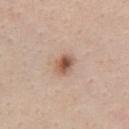Part of a total-body skin-imaging series; this lesion was reviewed on a skin check and was not flagged for biopsy.
The subject is a female aged 33 to 37.
Cropped from a whole-body photographic skin survey; the tile spans about 15 mm.
The lesion-visualizer software estimated a footprint of about 5 mm², an eccentricity of roughly 0.6, and a shape-asymmetry score of about 0.2 (0 = symmetric). The software also gave a lesion color around L≈56 a*≈20 b*≈29 in CIELAB, about 13 CIELAB-L* units darker than the surrounding skin, and a normalized lesion–skin contrast near 9. It also reported a border-irregularity rating of about 2/10, a within-lesion color-variation index near 7.5/10, and peripheral color asymmetry of about 2.
This is a white-light tile.
Located on the chest.
Measured at roughly 2.5 mm in maximum diameter.